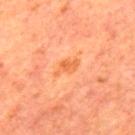Background: Cropped from a whole-body photographic skin survey; the tile spans about 15 mm. From the mid back. An algorithmic analysis of the crop reported a lesion–skin lightness drop of about 7 and a normalized lesion–skin contrast near 6. The software also gave a nevus-likeness score of about 0/100. A male patient, approximately 65 years of age. The recorded lesion diameter is about 3 mm. Captured under cross-polarized illumination.